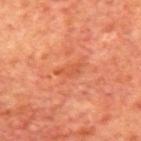image:
  source: total-body photography crop
  field_of_view_mm: 15
patient:
  sex: male
  age_approx: 70
lesion_size:
  long_diameter_mm_approx: 3.0
site: mid back